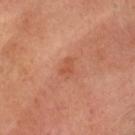Assessment:
The lesion was photographed on a routine skin check and not biopsied; there is no pathology result.
Image and clinical context:
The lesion-visualizer software estimated an average lesion color of about L≈52 a*≈28 b*≈35 (CIELAB) and a lesion–skin lightness drop of about 7. The analysis additionally found a classifier nevus-likeness of about 5/100 and a detector confidence of about 100 out of 100 that the crop contains a lesion. A region of skin cropped from a whole-body photographic capture, roughly 15 mm wide. Imaged with cross-polarized lighting. The recorded lesion diameter is about 2.5 mm. On the head or neck. The patient is roughly 55 years of age.